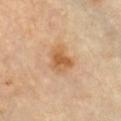Part of a total-body skin-imaging series; this lesion was reviewed on a skin check and was not flagged for biopsy. Imaged with cross-polarized lighting. On the chest. Approximately 3.5 mm at its widest. A lesion tile, about 15 mm wide, cut from a 3D total-body photograph. The subject is a female roughly 60 years of age.